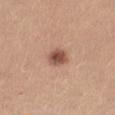follow-up=catalogued during a skin exam; not biopsied | patient=female, about 25 years old | tile lighting=white-light illumination | lesion diameter=about 3 mm | image=~15 mm crop, total-body skin-cancer survey | image-analysis metrics=a nevus-likeness score of about 95/100 and a detector confidence of about 100 out of 100 that the crop contains a lesion | location=the right thigh.